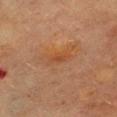Impression: The lesion was tiled from a total-body skin photograph and was not biopsied. Background: A 15 mm close-up tile from a total-body photography series done for melanoma screening. Located on the chest. A female subject, aged around 60.